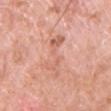Assessment:
Imaged during a routine full-body skin examination; the lesion was not biopsied and no histopathology is available.
Context:
Cropped from a total-body skin-imaging series; the visible field is about 15 mm. The lesion is located on the front of the torso. The subject is a male roughly 50 years of age. An algorithmic analysis of the crop reported a mean CIELAB color near L≈63 a*≈25 b*≈31, a lesion–skin lightness drop of about 7, and a lesion-to-skin contrast of about 5 (normalized; higher = more distinct). The software also gave a color-variation rating of about 3.5/10 and peripheral color asymmetry of about 1. Measured at roughly 5.5 mm in maximum diameter. Captured under white-light illumination.